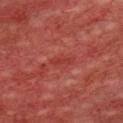workup = catalogued during a skin exam; not biopsied | image = 15 mm crop, total-body photography | subject = male, about 60 years old | lighting = cross-polarized illumination | automated metrics = a symmetry-axis asymmetry near 0.25; a border-irregularity rating of about 3/10 | location = the chest.